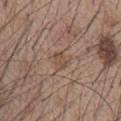The lesion was tiled from a total-body skin photograph and was not biopsied.
The lesion-visualizer software estimated an eccentricity of roughly 0.75 and a symmetry-axis asymmetry near 0.2. It also reported an average lesion color of about L≈48 a*≈16 b*≈27 (CIELAB).
A lesion tile, about 15 mm wide, cut from a 3D total-body photograph.
Imaged with white-light lighting.
Measured at roughly 2.5 mm in maximum diameter.
A male patient, roughly 55 years of age.
The lesion is located on the chest.